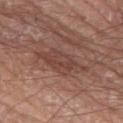Assessment: Captured during whole-body skin photography for melanoma surveillance; the lesion was not biopsied. Acquisition and patient details: The recorded lesion diameter is about 7 mm. A 15 mm close-up extracted from a 3D total-body photography capture. Captured under white-light illumination. The lesion-visualizer software estimated an area of roughly 12 mm², an eccentricity of roughly 0.95, and a shape-asymmetry score of about 0.3 (0 = symmetric). And it measured a border-irregularity rating of about 4.5/10 and a within-lesion color-variation index near 3/10. The subject is a male aged 78 to 82. The lesion is located on the right leg.